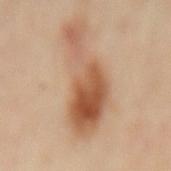Q: Was this lesion biopsied?
A: no biopsy performed (imaged during a skin exam)
Q: Lesion location?
A: the mid back
Q: Lesion size?
A: about 10.5 mm
Q: What did automated image analysis measure?
A: an eccentricity of roughly 0.95 and two-axis asymmetry of about 0.6; a lesion color around L≈55 a*≈21 b*≈34 in CIELAB and a normalized border contrast of about 9
Q: What is the imaging modality?
A: 15 mm crop, total-body photography
Q: Who is the patient?
A: female, aged 58–62